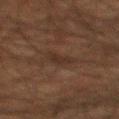This lesion was catalogued during total-body skin photography and was not selected for biopsy. The lesion is located on the mid back. A roughly 15 mm field-of-view crop from a total-body skin photograph. Measured at roughly 2.5 mm in maximum diameter. The subject is a male aged 58 to 62.